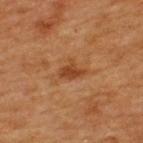Assessment:
Recorded during total-body skin imaging; not selected for excision or biopsy.
Background:
A 15 mm crop from a total-body photograph taken for skin-cancer surveillance. A female subject aged 38 to 42. The lesion is located on the upper back.